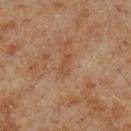workup = imaged on a skin check; not biopsied | patient = male, about 70 years old | body site = the leg | automated lesion analysis = a lesion color around L≈48 a*≈21 b*≈33 in CIELAB, roughly 5 lightness units darker than nearby skin, and a normalized lesion–skin contrast near 4.5; an automated nevus-likeness rating near 0 out of 100 and a lesion-detection confidence of about 100/100 | image = total-body-photography crop, ~15 mm field of view | lighting = cross-polarized illumination.